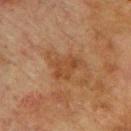The tile uses cross-polarized illumination. About 5 mm across. Cropped from a whole-body photographic skin survey; the tile spans about 15 mm. On the upper back. The patient is a male approximately 75 years of age.